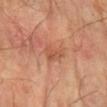No biopsy was performed on this lesion — it was imaged during a full skin examination and was not determined to be concerning.